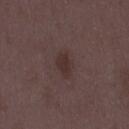Case summary:
• biopsy status · total-body-photography surveillance lesion; no biopsy
• image · ~15 mm tile from a whole-body skin photo
• patient · female, roughly 35 years of age
• location · the leg
• illumination · white-light
• TBP lesion metrics · an average lesion color of about L≈30 a*≈15 b*≈17 (CIELAB) and a normalized border contrast of about 7; a border-irregularity rating of about 2/10, a within-lesion color-variation index near 1.5/10, and radial color variation of about 0.5; lesion-presence confidence of about 100/100
• lesion diameter · ~3.5 mm (longest diameter)